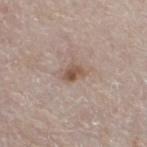{"biopsy_status": "not biopsied; imaged during a skin examination", "lesion_size": {"long_diameter_mm_approx": 2.5}, "image": {"source": "total-body photography crop", "field_of_view_mm": 15}, "site": "left lower leg", "automated_metrics": {"area_mm2_approx": 4.0, "shape_asymmetry": 0.3, "color_variation_0_10": 4.0, "peripheral_color_asymmetry": 1.5, "nevus_likeness_0_100": 60, "lesion_detection_confidence_0_100": 100}, "patient": {"sex": "male", "age_approx": 80}, "lighting": "white-light"}